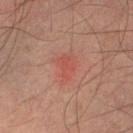Background: Approximately 3.5 mm at its widest. The lesion is located on the left lower leg. A male patient aged 43 to 47. Cropped from a total-body skin-imaging series; the visible field is about 15 mm. Automated image analysis of the tile measured a mean CIELAB color near L≈49 a*≈27 b*≈27, a lesion–skin lightness drop of about 5, and a normalized lesion–skin contrast near 4.5. The software also gave a color-variation rating of about 2/10 and peripheral color asymmetry of about 0.5. Captured under cross-polarized illumination.